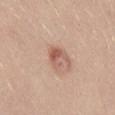Clinical impression: No biopsy was performed on this lesion — it was imaged during a full skin examination and was not determined to be concerning. Background: A 15 mm crop from a total-body photograph taken for skin-cancer surveillance. On the mid back. Captured under white-light illumination. Measured at roughly 2.5 mm in maximum diameter. A female subject roughly 40 years of age.